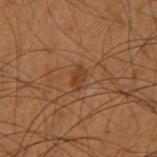Recorded during total-body skin imaging; not selected for excision or biopsy. Cropped from a whole-body photographic skin survey; the tile spans about 15 mm. A male subject, roughly 55 years of age. The recorded lesion diameter is about 2.5 mm. From the right forearm. The tile uses cross-polarized illumination.